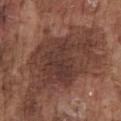The lesion was tiled from a total-body skin photograph and was not biopsied.
Automated image analysis of the tile measured an eccentricity of roughly 0.7 and a shape-asymmetry score of about 0.45 (0 = symmetric). The software also gave roughly 9 lightness units darker than nearby skin. The software also gave border irregularity of about 7.5 on a 0–10 scale and peripheral color asymmetry of about 1. It also reported an automated nevus-likeness rating near 0 out of 100 and lesion-presence confidence of about 95/100.
A 15 mm close-up tile from a total-body photography series done for melanoma screening.
Located on the chest.
A male patient, in their mid-70s.
The tile uses white-light illumination.